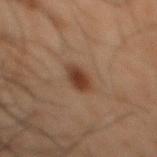Part of a total-body skin-imaging series; this lesion was reviewed on a skin check and was not flagged for biopsy. Approximately 3.5 mm at its widest. From the mid back. This image is a 15 mm lesion crop taken from a total-body photograph. A male patient, aged 48 to 52. The total-body-photography lesion software estimated a lesion color around L≈29 a*≈16 b*≈23 in CIELAB, about 9 CIELAB-L* units darker than the surrounding skin, and a normalized lesion–skin contrast near 9. It also reported an automated nevus-likeness rating near 100 out of 100 and lesion-presence confidence of about 100/100. The tile uses cross-polarized illumination.